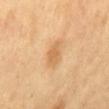biopsy status — catalogued during a skin exam; not biopsied
site — the mid back
patient — male, in their mid- to late 60s
lighting — cross-polarized illumination
image — 15 mm crop, total-body photography